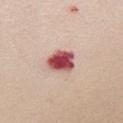Recorded during total-body skin imaging; not selected for excision or biopsy. About 4 mm across. From the mid back. The total-body-photography lesion software estimated a lesion area of about 8.5 mm², an eccentricity of roughly 0.7, and two-axis asymmetry of about 0.2. A female patient aged around 40. This is a white-light tile. This image is a 15 mm lesion crop taken from a total-body photograph.